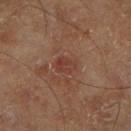Q: Is there a histopathology result?
A: no biopsy performed (imaged during a skin exam)
Q: Lesion size?
A: ~2.5 mm (longest diameter)
Q: What kind of image is this?
A: 15 mm crop, total-body photography
Q: How was the tile lit?
A: cross-polarized illumination
Q: Lesion location?
A: the right lower leg
Q: What are the patient's age and sex?
A: male, about 70 years old